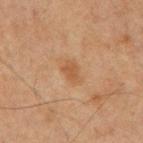biopsy_status: not biopsied; imaged during a skin examination
lesion_size:
  long_diameter_mm_approx: 3.0
automated_metrics:
  area_mm2_approx: 4.0
  shape_asymmetry: 0.3
  color_variation_0_10: 1.0
  peripheral_color_asymmetry: 0.5
image:
  source: total-body photography crop
  field_of_view_mm: 15
site: mid back
patient:
  sex: male
  age_approx: 65